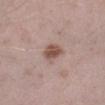Findings:
- follow-up — catalogued during a skin exam; not biopsied
- image — ~15 mm tile from a whole-body skin photo
- patient — male, about 40 years old
- body site — the left lower leg